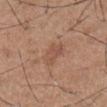Assessment:
Part of a total-body skin-imaging series; this lesion was reviewed on a skin check and was not flagged for biopsy.
Context:
Automated image analysis of the tile measured a mean CIELAB color near L≈52 a*≈21 b*≈29, a lesion–skin lightness drop of about 7, and a lesion-to-skin contrast of about 5 (normalized; higher = more distinct). And it measured a classifier nevus-likeness of about 0/100 and a detector confidence of about 100 out of 100 that the crop contains a lesion. Located on the abdomen. The subject is a male roughly 55 years of age. Measured at roughly 4 mm in maximum diameter. Captured under white-light illumination. This image is a 15 mm lesion crop taken from a total-body photograph.